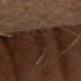Recorded during total-body skin imaging; not selected for excision or biopsy.
Longest diameter approximately 2.5 mm.
The total-body-photography lesion software estimated a lesion area of about 4.5 mm², an eccentricity of roughly 0.6, and a symmetry-axis asymmetry near 0.2. The analysis additionally found a lesion color around L≈14 a*≈11 b*≈16 in CIELAB, a lesion–skin lightness drop of about 4, and a normalized border contrast of about 6.5. And it measured a border-irregularity rating of about 1.5/10, a color-variation rating of about 1.5/10, and peripheral color asymmetry of about 0.5. And it measured a classifier nevus-likeness of about 0/100 and lesion-presence confidence of about 80/100.
A male subject roughly 80 years of age.
The lesion is on the left forearm.
A 15 mm close-up tile from a total-body photography series done for melanoma screening.
This is a cross-polarized tile.